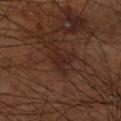Captured during whole-body skin photography for melanoma surveillance; the lesion was not biopsied.
A 15 mm close-up tile from a total-body photography series done for melanoma screening.
This is a cross-polarized tile.
An algorithmic analysis of the crop reported a lesion area of about 4 mm², an outline eccentricity of about 0.9 (0 = round, 1 = elongated), and a symmetry-axis asymmetry near 0.35. The software also gave an average lesion color of about L≈23 a*≈18 b*≈21 (CIELAB) and a normalized border contrast of about 7. The software also gave a color-variation rating of about 1.5/10 and a peripheral color-asymmetry measure near 0.5. The analysis additionally found an automated nevus-likeness rating near 0 out of 100 and a detector confidence of about 95 out of 100 that the crop contains a lesion.
Located on the right lower leg.
Approximately 3.5 mm at its widest.
The patient is a male aged 68–72.